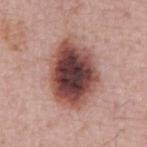Context:
On the front of the torso. About 8 mm across. The lesion-visualizer software estimated an area of roughly 32 mm², a shape eccentricity near 0.7, and a symmetry-axis asymmetry near 0.1. The analysis additionally found an automated nevus-likeness rating near 45 out of 100. A roughly 15 mm field-of-view crop from a total-body skin photograph. The patient is a male aged 63 to 67.